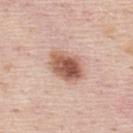This lesion was catalogued during total-body skin photography and was not selected for biopsy. A 15 mm close-up extracted from a 3D total-body photography capture. Automated image analysis of the tile measured border irregularity of about 2 on a 0–10 scale, internal color variation of about 6.5 on a 0–10 scale, and radial color variation of about 2. A male patient aged 43 to 47. The lesion's longest dimension is about 4.5 mm. The lesion is located on the mid back.